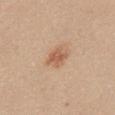biopsy status: catalogued during a skin exam; not biopsied
automated metrics: a lesion area of about 5 mm², an eccentricity of roughly 0.5, and two-axis asymmetry of about 0.2; an average lesion color of about L≈59 a*≈21 b*≈34 (CIELAB) and a normalized border contrast of about 7; a classifier nevus-likeness of about 85/100 and a detector confidence of about 100 out of 100 that the crop contains a lesion
site: the abdomen
image source: total-body-photography crop, ~15 mm field of view
patient: female, about 40 years old